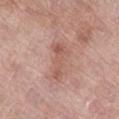Q: Is there a histopathology result?
A: total-body-photography surveillance lesion; no biopsy
Q: Who is the patient?
A: female, aged 58 to 62
Q: What is the anatomic site?
A: the right lower leg
Q: How was the tile lit?
A: white-light illumination
Q: What did automated image analysis measure?
A: a border-irregularity rating of about 7.5/10, a within-lesion color-variation index near 2/10, and radial color variation of about 0.5
Q: Lesion size?
A: ≈4.5 mm
Q: What is the imaging modality?
A: 15 mm crop, total-body photography